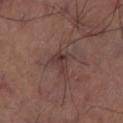Assessment: The lesion was photographed on a routine skin check and not biopsied; there is no pathology result. Clinical summary: The tile uses cross-polarized illumination. On the left thigh. The total-body-photography lesion software estimated a lesion color around L≈36 a*≈18 b*≈20 in CIELAB. The analysis additionally found a border-irregularity index near 5/10, internal color variation of about 4.5 on a 0–10 scale, and a peripheral color-asymmetry measure near 1.5. About 3 mm across. Cropped from a whole-body photographic skin survey; the tile spans about 15 mm.